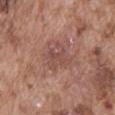Findings:
• biopsy status: no biopsy performed (imaged during a skin exam)
• image: ~15 mm tile from a whole-body skin photo
• automated metrics: a footprint of about 8.5 mm², an eccentricity of roughly 0.55, and a symmetry-axis asymmetry near 0.3; an automated nevus-likeness rating near 0 out of 100 and a detector confidence of about 100 out of 100 that the crop contains a lesion
• lighting: white-light
• anatomic site: the abdomen
• subject: male, aged around 75